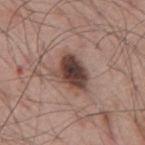– notes · no biopsy performed (imaged during a skin exam)
– image source · total-body-photography crop, ~15 mm field of view
– site · the back
– TBP lesion metrics · a classifier nevus-likeness of about 85/100 and lesion-presence confidence of about 100/100
– patient · male, about 55 years old
– diameter · ≈4.5 mm